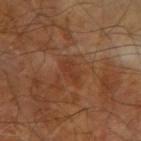  biopsy_status: not biopsied; imaged during a skin examination
  image:
    source: total-body photography crop
    field_of_view_mm: 15
  automated_metrics:
    cielab_L: 36
    cielab_a: 23
    cielab_b: 31
    vs_skin_darker_L: 6.0
    vs_skin_contrast_norm: 5.5
    border_irregularity_0_10: 3.0
    peripheral_color_asymmetry: 0.5
  site: right upper arm
  lesion_size:
    long_diameter_mm_approx: 3.0
  patient:
    sex: male
    age_approx: 70